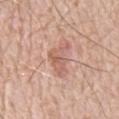The lesion was tiled from a total-body skin photograph and was not biopsied. A male subject aged around 80. Automated image analysis of the tile measured a lesion–skin lightness drop of about 9. The software also gave a nevus-likeness score of about 0/100 and lesion-presence confidence of about 100/100. The tile uses white-light illumination. The lesion is on the mid back. The lesion's longest dimension is about 4.5 mm. A 15 mm crop from a total-body photograph taken for skin-cancer surveillance.